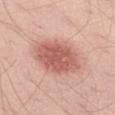Assessment:
Captured during whole-body skin photography for melanoma surveillance; the lesion was not biopsied.
Acquisition and patient details:
Located on the left thigh. A male patient, aged 48 to 52. A roughly 15 mm field-of-view crop from a total-body skin photograph. Captured under white-light illumination. Approximately 6 mm at its widest.